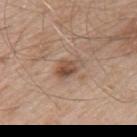Context: A male subject roughly 55 years of age. The lesion is located on the arm. A lesion tile, about 15 mm wide, cut from a 3D total-body photograph. Imaged with white-light lighting.